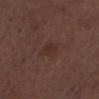Assessment: The lesion was tiled from a total-body skin photograph and was not biopsied. Clinical summary: Cropped from a total-body skin-imaging series; the visible field is about 15 mm. On the chest. A male patient, aged 73–77. Automated image analysis of the tile measured a mean CIELAB color near L≈29 a*≈17 b*≈21 and a normalized border contrast of about 5.5.